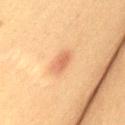workup: total-body-photography surveillance lesion; no biopsy
image: ~15 mm tile from a whole-body skin photo
subject: female, aged 48 to 52
lesion diameter: ~3 mm (longest diameter)
body site: the leg
image-analysis metrics: an outline eccentricity of about 0.8 (0 = round, 1 = elongated) and a shape-asymmetry score of about 0.2 (0 = symmetric); an average lesion color of about L≈56 a*≈22 b*≈34 (CIELAB) and about 9 CIELAB-L* units darker than the surrounding skin; a peripheral color-asymmetry measure near 0.5